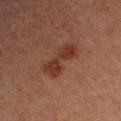• notes: imaged on a skin check; not biopsied
• location: the right upper arm
• lesion diameter: ≈4.5 mm
• image: ~15 mm crop, total-body skin-cancer survey
• subject: female, approximately 60 years of age